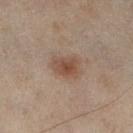<tbp_lesion>
<biopsy_status>not biopsied; imaged during a skin examination</biopsy_status>
<site>right lower leg</site>
<patient>
  <sex>male</sex>
  <age_approx>55</age_approx>
</patient>
<image>
  <source>total-body photography crop</source>
  <field_of_view_mm>15</field_of_view_mm>
</image>
<automated_metrics>
  <border_irregularity_0_10>2.5</border_irregularity_0_10>
  <peripheral_color_asymmetry>1.0</peripheral_color_asymmetry>
</automated_metrics>
</tbp_lesion>